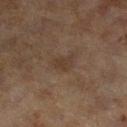notes: no biopsy performed (imaged during a skin exam) | patient: female, aged 58 to 62 | body site: the left lower leg | lesion diameter: ~2.5 mm (longest diameter) | illumination: cross-polarized | automated lesion analysis: a lesion area of about 4 mm², a shape eccentricity near 0.65, and a shape-asymmetry score of about 0.35 (0 = symmetric); a border-irregularity rating of about 3/10, a within-lesion color-variation index near 1.5/10, and peripheral color asymmetry of about 0.5; an automated nevus-likeness rating near 5 out of 100 and a detector confidence of about 100 out of 100 that the crop contains a lesion | imaging modality: 15 mm crop, total-body photography.